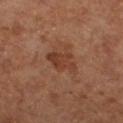Clinical impression: This lesion was catalogued during total-body skin photography and was not selected for biopsy. Context: The subject is a female aged around 65. On the left lower leg. A roughly 15 mm field-of-view crop from a total-body skin photograph.